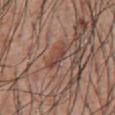No biopsy was performed on this lesion — it was imaged during a full skin examination and was not determined to be concerning. A male subject, roughly 55 years of age. Cropped from a whole-body photographic skin survey; the tile spans about 15 mm. The lesion's longest dimension is about 3.5 mm. The lesion-visualizer software estimated a lesion color around L≈44 a*≈21 b*≈26 in CIELAB, roughly 8 lightness units darker than nearby skin, and a normalized border contrast of about 6.5. The software also gave border irregularity of about 4 on a 0–10 scale, a within-lesion color-variation index near 0/10, and a peripheral color-asymmetry measure near 0. Captured under white-light illumination. The lesion is located on the abdomen.